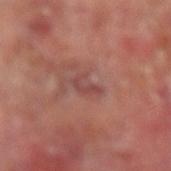follow-up: imaged on a skin check; not biopsied
TBP lesion metrics: two-axis asymmetry of about 0.5; a lesion color around L≈44 a*≈24 b*≈23 in CIELAB, about 7 CIELAB-L* units darker than the surrounding skin, and a lesion-to-skin contrast of about 5.5 (normalized; higher = more distinct)
illumination: cross-polarized
lesion diameter: ≈3.5 mm
subject: male, aged 63 to 67
acquisition: ~15 mm tile from a whole-body skin photo
location: the right forearm